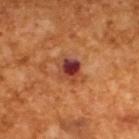{
  "biopsy_status": "not biopsied; imaged during a skin examination",
  "lesion_size": {
    "long_diameter_mm_approx": 2.5
  },
  "patient": {
    "sex": "male",
    "age_approx": 65
  },
  "lighting": "cross-polarized",
  "image": {
    "source": "total-body photography crop",
    "field_of_view_mm": 15
  }
}